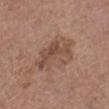workup: imaged on a skin check; not biopsied | imaging modality: 15 mm crop, total-body photography | illumination: white-light illumination | automated lesion analysis: a lesion–skin lightness drop of about 8 and a normalized border contrast of about 6; internal color variation of about 4.5 on a 0–10 scale and peripheral color asymmetry of about 1.5 | location: the abdomen | diameter: ≈6.5 mm | subject: female, roughly 65 years of age.